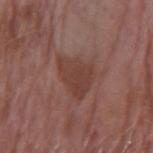Impression: This lesion was catalogued during total-body skin photography and was not selected for biopsy. Image and clinical context: Located on the arm. This is a white-light tile. A female patient approximately 70 years of age. Longest diameter approximately 5 mm. An algorithmic analysis of the crop reported a lesion area of about 12 mm² and an outline eccentricity of about 0.65 (0 = round, 1 = elongated). A 15 mm crop from a total-body photograph taken for skin-cancer surveillance.